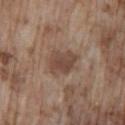Recorded during total-body skin imaging; not selected for excision or biopsy.
Imaged with white-light lighting.
A male subject roughly 75 years of age.
A close-up tile cropped from a whole-body skin photograph, about 15 mm across.
The lesion is located on the lower back.
Measured at roughly 3.5 mm in maximum diameter.